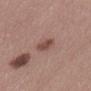notes: catalogued during a skin exam; not biopsied
tile lighting: white-light illumination
anatomic site: the mid back
subject: female, in their mid-40s
lesion diameter: ~3.5 mm (longest diameter)
image: 15 mm crop, total-body photography